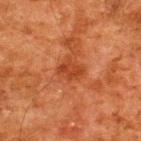The patient is a male roughly 60 years of age.
The tile uses cross-polarized illumination.
A 15 mm close-up extracted from a 3D total-body photography capture.
Located on the upper back.
The lesion-visualizer software estimated a lesion color around L≈34 a*≈27 b*≈33 in CIELAB, roughly 7 lightness units darker than nearby skin, and a normalized lesion–skin contrast near 6.5. The analysis additionally found a nevus-likeness score of about 0/100 and lesion-presence confidence of about 100/100.